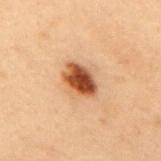{"biopsy_status": "not biopsied; imaged during a skin examination", "image": {"source": "total-body photography crop", "field_of_view_mm": 15}, "patient": {"sex": "male", "age_approx": 55}, "lesion_size": {"long_diameter_mm_approx": 4.0}, "site": "mid back"}